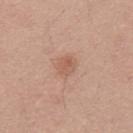Captured during whole-body skin photography for melanoma surveillance; the lesion was not biopsied.
On the mid back.
Cropped from a total-body skin-imaging series; the visible field is about 15 mm.
Captured under white-light illumination.
The subject is a male aged around 30.